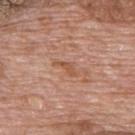Assessment: Imaged during a routine full-body skin examination; the lesion was not biopsied and no histopathology is available. Clinical summary: On the upper back. Cropped from a whole-body photographic skin survey; the tile spans about 15 mm. A male subject, roughly 70 years of age. This is a white-light tile.